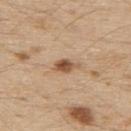Part of a total-body skin-imaging series; this lesion was reviewed on a skin check and was not flagged for biopsy. The lesion-visualizer software estimated a footprint of about 4 mm² and two-axis asymmetry of about 0.25. The software also gave a classifier nevus-likeness of about 95/100 and a lesion-detection confidence of about 100/100. This is a white-light tile. A lesion tile, about 15 mm wide, cut from a 3D total-body photograph. The lesion is on the upper back. Measured at roughly 3 mm in maximum diameter. A male patient, in their 70s.